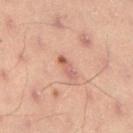The lesion was photographed on a routine skin check and not biopsied; there is no pathology result. Measured at roughly 2.5 mm in maximum diameter. On the left lower leg. Imaged with cross-polarized lighting. A female patient roughly 20 years of age. A 15 mm crop from a total-body photograph taken for skin-cancer surveillance. The lesion-visualizer software estimated an area of roughly 3 mm², an outline eccentricity of about 0.85 (0 = round, 1 = elongated), and a symmetry-axis asymmetry near 0.25. The analysis additionally found about 8 CIELAB-L* units darker than the surrounding skin and a normalized lesion–skin contrast near 6. And it measured a border-irregularity index near 2.5/10, a within-lesion color-variation index near 3/10, and peripheral color asymmetry of about 0.5. The software also gave a classifier nevus-likeness of about 10/100.